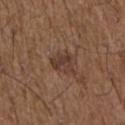follow-up = no biopsy performed (imaged during a skin exam); anatomic site = the left upper arm; image = ~15 mm crop, total-body skin-cancer survey; size = about 3 mm; lighting = white-light; subject = male, in their 50s.